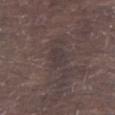<record>
  <automated_metrics>
    <area_mm2_approx>5.0</area_mm2_approx>
    <eccentricity>0.6</eccentricity>
    <shape_asymmetry>0.25</shape_asymmetry>
    <border_irregularity_0_10>2.5</border_irregularity_0_10>
    <peripheral_color_asymmetry>0.5</peripheral_color_asymmetry>
    <nevus_likeness_0_100>0</nevus_likeness_0_100>
    <lesion_detection_confidence_0_100>70</lesion_detection_confidence_0_100>
  </automated_metrics>
  <site>right lower leg</site>
  <patient>
    <sex>male</sex>
    <age_approx>70</age_approx>
  </patient>
  <image>
    <source>total-body photography crop</source>
    <field_of_view_mm>15</field_of_view_mm>
  </image>
</record>